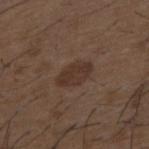Recorded during total-body skin imaging; not selected for excision or biopsy. Located on the mid back. A male subject aged approximately 50. Captured under white-light illumination. Approximately 4 mm at its widest. A roughly 15 mm field-of-view crop from a total-body skin photograph.